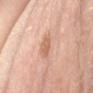{
  "biopsy_status": "not biopsied; imaged during a skin examination",
  "patient": {
    "sex": "male",
    "age_approx": 65
  },
  "lesion_size": {
    "long_diameter_mm_approx": 3.0
  },
  "site": "right forearm",
  "image": {
    "source": "total-body photography crop",
    "field_of_view_mm": 15
  }
}